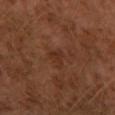Q: Was a biopsy performed?
A: no biopsy performed (imaged during a skin exam)
Q: Lesion location?
A: the left thigh
Q: What kind of image is this?
A: ~15 mm crop, total-body skin-cancer survey
Q: How large is the lesion?
A: ~2.5 mm (longest diameter)
Q: Patient demographics?
A: male, aged approximately 30
Q: What did automated image analysis measure?
A: a lesion–skin lightness drop of about 5 and a normalized border contrast of about 5.5; internal color variation of about 2 on a 0–10 scale and radial color variation of about 0.5